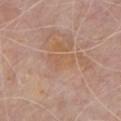{"biopsy_status": "not biopsied; imaged during a skin examination", "patient": {"sex": "male", "age_approx": 80}, "lesion_size": {"long_diameter_mm_approx": 3.5}, "image": {"source": "total-body photography crop", "field_of_view_mm": 15}, "automated_metrics": {"area_mm2_approx": 6.0, "eccentricity": 0.85, "shape_asymmetry": 0.4}, "lighting": "white-light", "site": "front of the torso"}